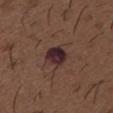The lesion was tiled from a total-body skin photograph and was not biopsied. On the chest. The patient is a male roughly 50 years of age. This image is a 15 mm lesion crop taken from a total-body photograph.